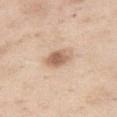Q: Was this lesion biopsied?
A: no biopsy performed (imaged during a skin exam)
Q: Who is the patient?
A: female, aged approximately 55
Q: What is the imaging modality?
A: 15 mm crop, total-body photography
Q: Where on the body is the lesion?
A: the leg
Q: What is the lesion's diameter?
A: ~3.5 mm (longest diameter)
Q: How was the tile lit?
A: white-light illumination